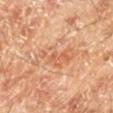follow-up: no biopsy performed (imaged during a skin exam)
location: the leg
automated metrics: an area of roughly 13 mm², an eccentricity of roughly 0.85, and two-axis asymmetry of about 0.4; an average lesion color of about L≈61 a*≈24 b*≈35 (CIELAB), a lesion–skin lightness drop of about 7, and a normalized border contrast of about 4.5; a lesion-detection confidence of about 100/100
image: 15 mm crop, total-body photography
patient: male, aged around 70
diameter: ≈5.5 mm